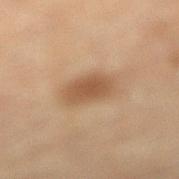The total-body-photography lesion software estimated a border-irregularity rating of about 2/10, a within-lesion color-variation index near 2/10, and peripheral color asymmetry of about 0.5. It also reported a nevus-likeness score of about 95/100 and a detector confidence of about 100 out of 100 that the crop contains a lesion. A female patient, in their mid- to late 50s. Cropped from a whole-body photographic skin survey; the tile spans about 15 mm. The lesion's longest dimension is about 4 mm. Captured under cross-polarized illumination. On the right lower leg.